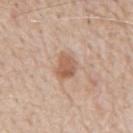The lesion was tiled from a total-body skin photograph and was not biopsied.
A male patient in their 80s.
The tile uses white-light illumination.
Cropped from a total-body skin-imaging series; the visible field is about 15 mm.
The lesion is located on the mid back.
Measured at roughly 3 mm in maximum diameter.
The total-body-photography lesion software estimated a lesion color around L≈58 a*≈20 b*≈31 in CIELAB and a normalized lesion–skin contrast near 7.5.